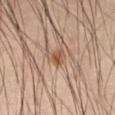The lesion is located on the abdomen.
The subject is a male about 55 years old.
A region of skin cropped from a whole-body photographic capture, roughly 15 mm wide.
This is a cross-polarized tile.
The lesion-visualizer software estimated a mean CIELAB color near L≈53 a*≈21 b*≈32 and about 10 CIELAB-L* units darker than the surrounding skin.